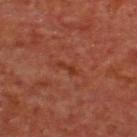  biopsy_status: not biopsied; imaged during a skin examination
  patient:
    sex: male
    age_approx: 65
  lesion_size:
    long_diameter_mm_approx: 2.5
  lighting: cross-polarized
  site: upper back
  automated_metrics:
    area_mm2_approx: 2.5
    eccentricity: 0.9
    shape_asymmetry: 0.4
    cielab_L: 32
    cielab_a: 25
    cielab_b: 30
    vs_skin_darker_L: 5.0
    vs_skin_contrast_norm: 5.5
    nevus_likeness_0_100: 0
  image:
    source: total-body photography crop
    field_of_view_mm: 15